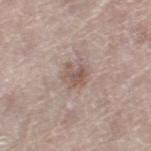Imaged during a routine full-body skin examination; the lesion was not biopsied and no histopathology is available.
A 15 mm crop from a total-body photograph taken for skin-cancer surveillance.
A female subject, aged 58 to 62.
Longest diameter approximately 2.5 mm.
Automated image analysis of the tile measured a mean CIELAB color near L≈54 a*≈16 b*≈22, a lesion–skin lightness drop of about 9, and a normalized lesion–skin contrast near 6.5. The analysis additionally found a border-irregularity index near 4/10, internal color variation of about 2.5 on a 0–10 scale, and radial color variation of about 0.5.
Located on the left thigh.
Captured under white-light illumination.